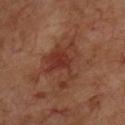subject = male, aged around 65; image = ~15 mm tile from a whole-body skin photo.